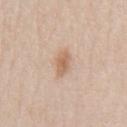The lesion was tiled from a total-body skin photograph and was not biopsied.